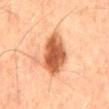{"biopsy_status": "not biopsied; imaged during a skin examination", "image": {"source": "total-body photography crop", "field_of_view_mm": 15}, "lighting": "cross-polarized", "site": "mid back", "lesion_size": {"long_diameter_mm_approx": 6.5}, "patient": {"sex": "male", "age_approx": 65}}